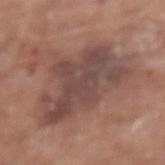biopsy status — no biopsy performed (imaged during a skin exam) | image-analysis metrics — a lesion area of about 37 mm² and a shape-asymmetry score of about 0.35 (0 = symmetric); an average lesion color of about L≈46 a*≈18 b*≈21 (CIELAB), about 10 CIELAB-L* units darker than the surrounding skin, and a normalized border contrast of about 8; a border-irregularity index near 5.5/10, internal color variation of about 5 on a 0–10 scale, and radial color variation of about 1.5; an automated nevus-likeness rating near 0 out of 100 and a detector confidence of about 100 out of 100 that the crop contains a lesion | patient — female, about 75 years old | site — the upper back | image — 15 mm crop, total-body photography | lesion size — ~9.5 mm (longest diameter).